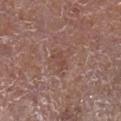<tbp_lesion>
<lighting>white-light</lighting>
<image>
  <source>total-body photography crop</source>
  <field_of_view_mm>15</field_of_view_mm>
</image>
<patient>
  <sex>male</sex>
  <age_approx>75</age_approx>
</patient>
<site>leg</site>
<automated_metrics>
  <area_mm2_approx>3.0</area_mm2_approx>
  <eccentricity>0.85</eccentricity>
  <shape_asymmetry>0.35</shape_asymmetry>
  <peripheral_color_asymmetry>0.0</peripheral_color_asymmetry>
</automated_metrics>
<lesion_size>
  <long_diameter_mm_approx>2.5</long_diameter_mm_approx>
</lesion_size>
</tbp_lesion>